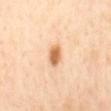biopsy status = imaged on a skin check; not biopsied | image = 15 mm crop, total-body photography | anatomic site = the mid back | diameter = ~3 mm (longest diameter) | patient = female, aged 38 to 42.